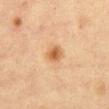Impression:
The lesion was tiled from a total-body skin photograph and was not biopsied.
Clinical summary:
The subject is a female roughly 40 years of age. On the front of the torso. A 15 mm crop from a total-body photograph taken for skin-cancer surveillance. Approximately 2.5 mm at its widest. The tile uses cross-polarized illumination.